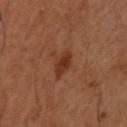Assessment:
Part of a total-body skin-imaging series; this lesion was reviewed on a skin check and was not flagged for biopsy.
Image and clinical context:
The patient is a male roughly 55 years of age. Located on the upper back. Imaged with cross-polarized lighting. A lesion tile, about 15 mm wide, cut from a 3D total-body photograph. Approximately 3.5 mm at its widest.